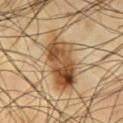Clinical impression:
The lesion was tiled from a total-body skin photograph and was not biopsied.
Acquisition and patient details:
A male subject, aged 58–62. This image is a 15 mm lesion crop taken from a total-body photograph. Located on the front of the torso.